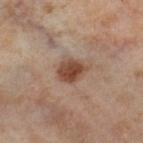– biopsy status: total-body-photography surveillance lesion; no biopsy
– acquisition: total-body-photography crop, ~15 mm field of view
– size: ≈3 mm
– tile lighting: cross-polarized
– site: the right thigh
– patient: female, about 55 years old